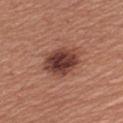| field | value |
|---|---|
| biopsy status | imaged on a skin check; not biopsied |
| size | ~5 mm (longest diameter) |
| patient | female, roughly 55 years of age |
| body site | the left upper arm |
| tile lighting | white-light illumination |
| imaging modality | total-body-photography crop, ~15 mm field of view |